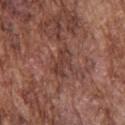The lesion was photographed on a routine skin check and not biopsied; there is no pathology result.
The subject is a male aged around 75.
A 15 mm crop from a total-body photograph taken for skin-cancer surveillance.
The lesion-visualizer software estimated a lesion color around L≈39 a*≈22 b*≈25 in CIELAB and a lesion–skin lightness drop of about 7. And it measured a border-irregularity index near 5/10, a color-variation rating of about 2/10, and peripheral color asymmetry of about 0.5. And it measured a nevus-likeness score of about 0/100 and a detector confidence of about 70 out of 100 that the crop contains a lesion.
Imaged with white-light lighting.
The recorded lesion diameter is about 4 mm.
On the upper back.